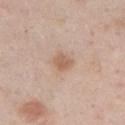The lesion was tiled from a total-body skin photograph and was not biopsied. A male subject aged approximately 50. A roughly 15 mm field-of-view crop from a total-body skin photograph. The lesion is located on the chest.